A female patient, aged around 40 · a lesion tile, about 15 mm wide, cut from a 3D total-body photograph · the lesion is located on the right thigh — 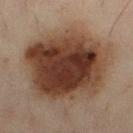Findings:
– lesion size · about 9.5 mm
– lighting · cross-polarized
– TBP lesion metrics · a lesion area of about 60 mm² and an eccentricity of roughly 0.55; an average lesion color of about L≈34 a*≈17 b*≈24 (CIELAB), a lesion–skin lightness drop of about 15, and a normalized border contrast of about 13; border irregularity of about 2 on a 0–10 scale, a color-variation rating of about 7.5/10, and peripheral color asymmetry of about 2.5
– histopathology · an intradermal melanocytic nevus